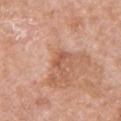Part of a total-body skin-imaging series; this lesion was reviewed on a skin check and was not flagged for biopsy. From the front of the torso. The subject is a female approximately 60 years of age. A 15 mm close-up extracted from a 3D total-body photography capture.